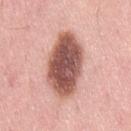follow-up = total-body-photography surveillance lesion; no biopsy | anatomic site = the lower back | tile lighting = white-light illumination | image source = ~15 mm crop, total-body skin-cancer survey | lesion diameter = ~7.5 mm (longest diameter) | patient = female, approximately 50 years of age.